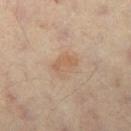Q: Is there a histopathology result?
A: imaged on a skin check; not biopsied
Q: What kind of image is this?
A: total-body-photography crop, ~15 mm field of view
Q: Where on the body is the lesion?
A: the right thigh
Q: Who is the patient?
A: male, in their 60s
Q: How was the tile lit?
A: cross-polarized